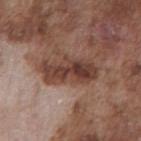Assessment:
The lesion was photographed on a routine skin check and not biopsied; there is no pathology result.
Acquisition and patient details:
A 15 mm crop from a total-body photograph taken for skin-cancer surveillance. The lesion-visualizer software estimated border irregularity of about 4.5 on a 0–10 scale, a within-lesion color-variation index near 6.5/10, and radial color variation of about 2.5. And it measured lesion-presence confidence of about 90/100. Captured under white-light illumination. Measured at roughly 6 mm in maximum diameter. A male patient about 75 years old. Located on the chest.